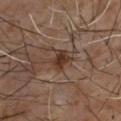Recorded during total-body skin imaging; not selected for excision or biopsy.
The total-body-photography lesion software estimated an area of roughly 4.5 mm² and an eccentricity of roughly 0.8. The analysis additionally found a border-irregularity rating of about 3.5/10 and a color-variation rating of about 2.5/10.
A male subject aged approximately 60.
A close-up tile cropped from a whole-body skin photograph, about 15 mm across.
Captured under cross-polarized illumination.
The recorded lesion diameter is about 3 mm.
Located on the chest.